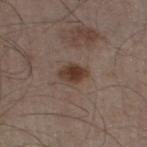Assessment: No biopsy was performed on this lesion — it was imaged during a full skin examination and was not determined to be concerning. Clinical summary: The subject is a male aged 58–62. Located on the left thigh. The lesion-visualizer software estimated an automated nevus-likeness rating near 95 out of 100 and a detector confidence of about 100 out of 100 that the crop contains a lesion. Imaged with white-light lighting. Approximately 3 mm at its widest. A region of skin cropped from a whole-body photographic capture, roughly 15 mm wide.